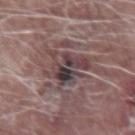The lesion was photographed on a routine skin check and not biopsied; there is no pathology result. A male subject, aged 63–67. Captured under white-light illumination. The lesion is located on the right forearm. The recorded lesion diameter is about 6 mm. Cropped from a total-body skin-imaging series; the visible field is about 15 mm.